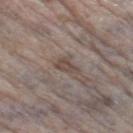Assessment:
The lesion was photographed on a routine skin check and not biopsied; there is no pathology result.
Acquisition and patient details:
Cropped from a total-body skin-imaging series; the visible field is about 15 mm. A female patient, about 85 years old or older. The lesion is located on the right thigh.